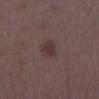workup: total-body-photography surveillance lesion; no biopsy | acquisition: ~15 mm tile from a whole-body skin photo | subject: female, approximately 35 years of age | body site: the right lower leg.